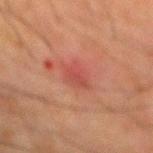Assessment: Recorded during total-body skin imaging; not selected for excision or biopsy. Context: A male subject aged 63 to 67. Located on the mid back. This image is a 15 mm lesion crop taken from a total-body photograph.